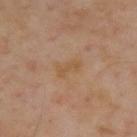Q: Was this lesion biopsied?
A: catalogued during a skin exam; not biopsied
Q: What is the anatomic site?
A: the upper back
Q: Patient demographics?
A: male, aged 53 to 57
Q: What kind of image is this?
A: ~15 mm crop, total-body skin-cancer survey